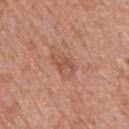The lesion was photographed on a routine skin check and not biopsied; there is no pathology result. The patient is a male in their 70s. Automated image analysis of the tile measured a footprint of about 6 mm², an eccentricity of roughly 0.75, and two-axis asymmetry of about 0.25. And it measured an average lesion color of about L≈53 a*≈24 b*≈30 (CIELAB), about 8 CIELAB-L* units darker than the surrounding skin, and a normalized lesion–skin contrast near 5.5. The lesion is on the upper back. A 15 mm crop from a total-body photograph taken for skin-cancer surveillance. Approximately 3.5 mm at its widest. Captured under white-light illumination.